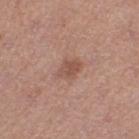Impression:
Captured during whole-body skin photography for melanoma surveillance; the lesion was not biopsied.
Clinical summary:
A female patient approximately 35 years of age. A 15 mm close-up tile from a total-body photography series done for melanoma screening. The lesion is located on the leg.